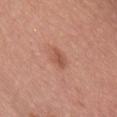TBP lesion metrics — an average lesion color of about L≈54 a*≈25 b*≈31 (CIELAB) and a normalized border contrast of about 6; a border-irregularity index near 2.5/10 | location — the chest | lesion diameter — ≈3 mm | image source — 15 mm crop, total-body photography | subject — female, aged 38 to 42.